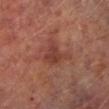  biopsy_status: not biopsied; imaged during a skin examination
  image:
    source: total-body photography crop
    field_of_view_mm: 15
  patient:
    sex: male
    age_approx: 65
  lighting: cross-polarized
  lesion_size:
    long_diameter_mm_approx: 3.5
  site: left lower leg
  automated_metrics:
    cielab_L: 39
    cielab_a: 25
    cielab_b: 26
    vs_skin_darker_L: 8.0
    border_irregularity_0_10: 4.5
    color_variation_0_10: 3.0
    peripheral_color_asymmetry: 1.0
    nevus_likeness_0_100: 0
    lesion_detection_confidence_0_100: 100